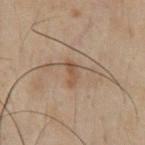Recorded during total-body skin imaging; not selected for excision or biopsy. A male patient aged approximately 50. The lesion is located on the front of the torso. The tile uses cross-polarized illumination. About 3 mm across. A 15 mm close-up extracted from a 3D total-body photography capture.